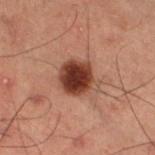notes = imaged on a skin check; not biopsied
subject = male, aged approximately 60
illumination = cross-polarized
automated lesion analysis = an average lesion color of about L≈30 a*≈19 b*≈23 (CIELAB) and roughly 14 lightness units darker than nearby skin; a border-irregularity rating of about 2/10, a within-lesion color-variation index near 4.5/10, and peripheral color asymmetry of about 1; a classifier nevus-likeness of about 100/100 and lesion-presence confidence of about 100/100
site = the right thigh
image source = total-body-photography crop, ~15 mm field of view
lesion diameter = ≈4.5 mm